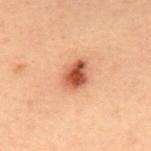Notes:
- follow-up: total-body-photography surveillance lesion; no biopsy
- automated metrics: a border-irregularity index near 2/10, a within-lesion color-variation index near 7/10, and radial color variation of about 2.5; a nevus-likeness score of about 100/100 and a lesion-detection confidence of about 100/100
- image: ~15 mm tile from a whole-body skin photo
- subject: male, roughly 55 years of age
- anatomic site: the upper back
- lesion diameter: about 3.5 mm